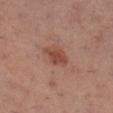No biopsy was performed on this lesion — it was imaged during a full skin examination and was not determined to be concerning.
The recorded lesion diameter is about 3.5 mm.
From the right lower leg.
A 15 mm crop from a total-body photograph taken for skin-cancer surveillance.
This is a white-light tile.
Automated tile analysis of the lesion measured a lesion color around L≈47 a*≈23 b*≈29 in CIELAB, about 9 CIELAB-L* units darker than the surrounding skin, and a normalized border contrast of about 7.5. The analysis additionally found a border-irregularity index near 2.5/10, a within-lesion color-variation index near 2.5/10, and a peripheral color-asymmetry measure near 1. The software also gave a classifier nevus-likeness of about 55/100 and a detector confidence of about 100 out of 100 that the crop contains a lesion.
The patient is a female aged 28–32.